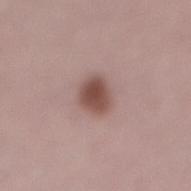workup — total-body-photography surveillance lesion; no biopsy | imaging modality — total-body-photography crop, ~15 mm field of view | automated metrics — an average lesion color of about L≈49 a*≈20 b*≈22 (CIELAB), about 13 CIELAB-L* units darker than the surrounding skin, and a normalized border contrast of about 9.5; border irregularity of about 1.5 on a 0–10 scale, a within-lesion color-variation index near 3/10, and a peripheral color-asymmetry measure near 1; an automated nevus-likeness rating near 100 out of 100 | tile lighting — white-light illumination | patient — male, roughly 70 years of age | lesion diameter — ≈3.5 mm | location — the lower back.